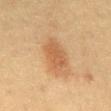Q: Is there a histopathology result?
A: no biopsy performed (imaged during a skin exam)
Q: Lesion location?
A: the mid back
Q: What are the patient's age and sex?
A: female, approximately 50 years of age
Q: What is the lesion's diameter?
A: about 4.5 mm
Q: What kind of image is this?
A: total-body-photography crop, ~15 mm field of view
Q: Automated lesion metrics?
A: a shape eccentricity near 0.75 and a shape-asymmetry score of about 0.2 (0 = symmetric); an average lesion color of about L≈49 a*≈19 b*≈33 (CIELAB), roughly 8 lightness units darker than nearby skin, and a lesion-to-skin contrast of about 6.5 (normalized; higher = more distinct); a nevus-likeness score of about 100/100 and lesion-presence confidence of about 100/100
Q: Illumination type?
A: cross-polarized